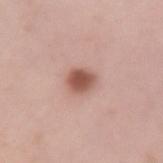biopsy_status: not biopsied; imaged during a skin examination
site: left upper arm
image:
  source: total-body photography crop
  field_of_view_mm: 15
lesion_size:
  long_diameter_mm_approx: 3.0
automated_metrics:
  vs_skin_darker_L: 15.0
  vs_skin_contrast_norm: 9.5
  nevus_likeness_0_100: 100
patient:
  sex: female
  age_approx: 40